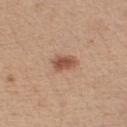biopsy status = no biopsy performed (imaged during a skin exam).